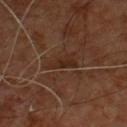| feature | finding |
|---|---|
| notes | no biopsy performed (imaged during a skin exam) |
| automated metrics | a lesion area of about 4.5 mm², an eccentricity of roughly 0.9, and two-axis asymmetry of about 0.45; a border-irregularity rating of about 4.5/10, internal color variation of about 2 on a 0–10 scale, and peripheral color asymmetry of about 0.5 |
| site | the chest |
| acquisition | ~15 mm tile from a whole-body skin photo |
| lighting | cross-polarized illumination |
| diameter | ~3.5 mm (longest diameter) |
| patient | male, roughly 55 years of age |